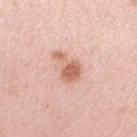biopsy status: no biopsy performed (imaged during a skin exam)
lesion diameter: ≈4 mm
acquisition: ~15 mm tile from a whole-body skin photo
anatomic site: the arm
automated metrics: roughly 12 lightness units darker than nearby skin and a normalized border contrast of about 7.5; border irregularity of about 5 on a 0–10 scale and peripheral color asymmetry of about 1
patient: female, roughly 40 years of age
illumination: white-light illumination